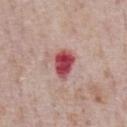| key | value |
|---|---|
| workup | no biopsy performed (imaged during a skin exam) |
| imaging modality | ~15 mm tile from a whole-body skin photo |
| subject | male, aged 73 to 77 |
| lesion diameter | about 3.5 mm |
| anatomic site | the chest |
| tile lighting | white-light |